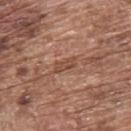The lesion is located on the upper back.
About 3 mm across.
A male patient, in their mid- to late 70s.
A lesion tile, about 15 mm wide, cut from a 3D total-body photograph.
Imaged with white-light lighting.